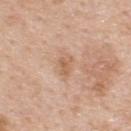Q: Was this lesion biopsied?
A: imaged on a skin check; not biopsied
Q: How large is the lesion?
A: about 2.5 mm
Q: What are the patient's age and sex?
A: male, aged around 50
Q: Lesion location?
A: the upper back
Q: How was the tile lit?
A: white-light illumination
Q: What is the imaging modality?
A: ~15 mm crop, total-body skin-cancer survey
Q: What did automated image analysis measure?
A: a mean CIELAB color near L≈60 a*≈20 b*≈33, a lesion–skin lightness drop of about 8, and a normalized lesion–skin contrast near 6